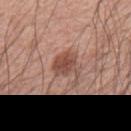Clinical summary: The tile uses white-light illumination. From the back. Automated image analysis of the tile measured border irregularity of about 4 on a 0–10 scale, a within-lesion color-variation index near 3.5/10, and radial color variation of about 1. The patient is a male in their mid- to late 50s. A region of skin cropped from a whole-body photographic capture, roughly 15 mm wide. The recorded lesion diameter is about 3.5 mm.